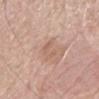Background: The lesion's longest dimension is about 3 mm. Imaged with white-light lighting. From the mid back. The lesion-visualizer software estimated a mean CIELAB color near L≈60 a*≈19 b*≈28, about 7 CIELAB-L* units darker than the surrounding skin, and a normalized lesion–skin contrast near 4.5. It also reported a border-irregularity rating of about 6.5/10, a color-variation rating of about 0/10, and peripheral color asymmetry of about 0. The software also gave a nevus-likeness score of about 0/100 and a detector confidence of about 80 out of 100 that the crop contains a lesion. A male patient, aged around 80. A roughly 15 mm field-of-view crop from a total-body skin photograph.